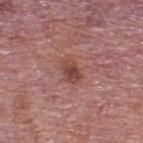Imaged during a routine full-body skin examination; the lesion was not biopsied and no histopathology is available.
This image is a 15 mm lesion crop taken from a total-body photograph.
The subject is a male in their mid-70s.
Imaged with white-light lighting.
The lesion is located on the back.